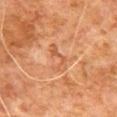<tbp_lesion>
  <biopsy_status>not biopsied; imaged during a skin examination</biopsy_status>
  <lighting>cross-polarized</lighting>
  <site>chest</site>
  <image>
    <source>total-body photography crop</source>
    <field_of_view_mm>15</field_of_view_mm>
  </image>
  <patient>
    <sex>male</sex>
    <age_approx>80</age_approx>
  </patient>
  <lesion_size>
    <long_diameter_mm_approx>3.5</long_diameter_mm_approx>
  </lesion_size>
</tbp_lesion>